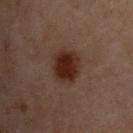workup: catalogued during a skin exam; not biopsied | anatomic site: the upper back | image: 15 mm crop, total-body photography | TBP lesion metrics: a within-lesion color-variation index near 3.5/10; a classifier nevus-likeness of about 100/100 and a lesion-detection confidence of about 100/100 | patient: male, approximately 50 years of age | tile lighting: cross-polarized illumination.